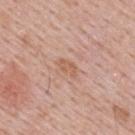Recorded during total-body skin imaging; not selected for excision or biopsy.
A male subject, about 40 years old.
The recorded lesion diameter is about 3 mm.
A region of skin cropped from a whole-body photographic capture, roughly 15 mm wide.
This is a white-light tile.
Automated image analysis of the tile measured an average lesion color of about L≈59 a*≈21 b*≈31 (CIELAB), about 7 CIELAB-L* units darker than the surrounding skin, and a lesion-to-skin contrast of about 5.5 (normalized; higher = more distinct). And it measured an automated nevus-likeness rating near 0 out of 100 and a detector confidence of about 100 out of 100 that the crop contains a lesion.
From the mid back.